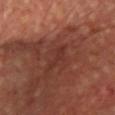{"lighting": "cross-polarized", "image": {"source": "total-body photography crop", "field_of_view_mm": 15}, "site": "front of the torso", "patient": {"age_approx": 65}}